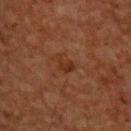• image source: total-body-photography crop, ~15 mm field of view
• patient: male, roughly 50 years of age
• anatomic site: the back
• diameter: ~2.5 mm (longest diameter)